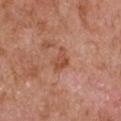No biopsy was performed on this lesion — it was imaged during a full skin examination and was not determined to be concerning. Automated image analysis of the tile measured a lesion color around L≈51 a*≈25 b*≈33 in CIELAB, roughly 9 lightness units darker than nearby skin, and a normalized lesion–skin contrast near 7. The software also gave a border-irregularity rating of about 3/10, a within-lesion color-variation index near 2/10, and radial color variation of about 0.5. The software also gave a classifier nevus-likeness of about 0/100 and a lesion-detection confidence of about 100/100. A male subject in their mid-60s. From the left upper arm. Captured under white-light illumination. A 15 mm close-up tile from a total-body photography series done for melanoma screening. Measured at roughly 3 mm in maximum diameter.